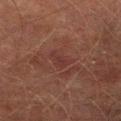The lesion was tiled from a total-body skin photograph and was not biopsied. A close-up tile cropped from a whole-body skin photograph, about 15 mm across. Automated image analysis of the tile measured a lesion color around L≈28 a*≈20 b*≈19 in CIELAB, about 4 CIELAB-L* units darker than the surrounding skin, and a normalized lesion–skin contrast near 5. The subject is a male aged around 75. Measured at roughly 3 mm in maximum diameter. The lesion is located on the leg. This is a cross-polarized tile.